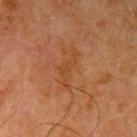This lesion was catalogued during total-body skin photography and was not selected for biopsy.
This is a cross-polarized tile.
A close-up tile cropped from a whole-body skin photograph, about 15 mm across.
Approximately 4.5 mm at its widest.
The total-body-photography lesion software estimated a lesion color around L≈35 a*≈20 b*≈31 in CIELAB, a lesion–skin lightness drop of about 5, and a normalized lesion–skin contrast near 5. The software also gave a within-lesion color-variation index near 0.5/10.
A male patient, aged 78 to 82.
Located on the left upper arm.